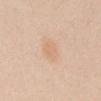{
  "biopsy_status": "not biopsied; imaged during a skin examination",
  "automated_metrics": {
    "border_irregularity_0_10": 2.5,
    "peripheral_color_asymmetry": 1.0
  },
  "image": {
    "source": "total-body photography crop",
    "field_of_view_mm": 15
  },
  "lighting": "white-light",
  "lesion_size": {
    "long_diameter_mm_approx": 2.5
  },
  "site": "abdomen",
  "patient": {
    "sex": "female",
    "age_approx": 20
  }
}